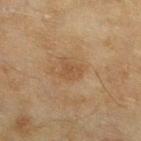biopsy status = no biopsy performed (imaged during a skin exam); size = ~3 mm (longest diameter); location = the left lower leg; patient = female, about 60 years old; imaging modality = total-body-photography crop, ~15 mm field of view.